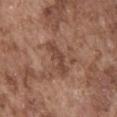| field | value |
|---|---|
| workup | catalogued during a skin exam; not biopsied |
| automated lesion analysis | an area of roughly 6.5 mm², an outline eccentricity of about 0.95 (0 = round, 1 = elongated), and a shape-asymmetry score of about 0.45 (0 = symmetric); a border-irregularity index near 5.5/10, internal color variation of about 1 on a 0–10 scale, and peripheral color asymmetry of about 0.5; a nevus-likeness score of about 0/100 and a detector confidence of about 90 out of 100 that the crop contains a lesion |
| illumination | white-light |
| subject | male, about 75 years old |
| image source | total-body-photography crop, ~15 mm field of view |
| anatomic site | the abdomen |
| size | ~5 mm (longest diameter) |